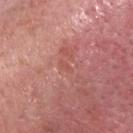Captured during whole-body skin photography for melanoma surveillance; the lesion was not biopsied. Located on the head or neck. Imaged with white-light lighting. The lesion's longest dimension is about 1.5 mm. The subject is a female aged around 60. A 15 mm crop from a total-body photograph taken for skin-cancer surveillance.